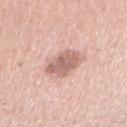Recorded during total-body skin imaging; not selected for excision or biopsy.
A female subject in their mid-50s.
Imaged with white-light lighting.
Automated tile analysis of the lesion measured an average lesion color of about L≈63 a*≈22 b*≈24 (CIELAB) and roughly 13 lightness units darker than nearby skin. The software also gave a border-irregularity index near 2/10 and a color-variation rating of about 3/10.
The lesion's longest dimension is about 4 mm.
From the right upper arm.
A 15 mm close-up extracted from a 3D total-body photography capture.